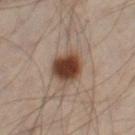follow-up: imaged on a skin check; not biopsied
subject: male, aged around 50
image: 15 mm crop, total-body photography
automated lesion analysis: a shape eccentricity near 0.5
site: the left leg
illumination: cross-polarized illumination
lesion diameter: ~4 mm (longest diameter)